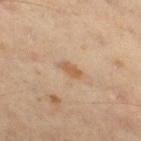Recorded during total-body skin imaging; not selected for excision or biopsy.
A region of skin cropped from a whole-body photographic capture, roughly 15 mm wide.
The lesion is located on the right thigh.
Measured at roughly 3 mm in maximum diameter.
A male subject, about 60 years old.
This is a cross-polarized tile.